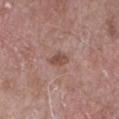A 15 mm crop from a total-body photograph taken for skin-cancer surveillance. The tile uses white-light illumination. Approximately 2.5 mm at its widest. The lesion is located on the right forearm. The subject is a male roughly 60 years of age.